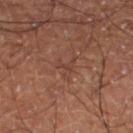A lesion tile, about 15 mm wide, cut from a 3D total-body photograph.
The patient is a male aged 58–62.
The lesion is on the left lower leg.
The tile uses cross-polarized illumination.
The lesion-visualizer software estimated a border-irregularity rating of about 8.5/10, internal color variation of about 0 on a 0–10 scale, and a peripheral color-asymmetry measure near 0.
About 3 mm across.